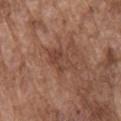Assessment:
The lesion was tiled from a total-body skin photograph and was not biopsied.
Background:
On the chest. A 15 mm close-up extracted from a 3D total-body photography capture. Automated tile analysis of the lesion measured a shape-asymmetry score of about 0.35 (0 = symmetric). And it measured a border-irregularity rating of about 4.5/10, internal color variation of about 4.5 on a 0–10 scale, and a peripheral color-asymmetry measure near 1.5. The software also gave a classifier nevus-likeness of about 0/100. Captured under white-light illumination. The subject is a male roughly 75 years of age.